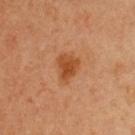The total-body-photography lesion software estimated a shape eccentricity near 0.65. It also reported a border-irregularity index near 3/10, internal color variation of about 2.5 on a 0–10 scale, and a peripheral color-asymmetry measure near 1. It also reported an automated nevus-likeness rating near 90 out of 100 and lesion-presence confidence of about 100/100. Longest diameter approximately 3.5 mm. A female subject aged 48 to 52. Imaged with cross-polarized lighting. This image is a 15 mm lesion crop taken from a total-body photograph.